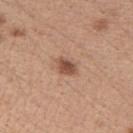The lesion was tiled from a total-body skin photograph and was not biopsied.
This is a white-light tile.
From the right upper arm.
A 15 mm close-up extracted from a 3D total-body photography capture.
The subject is a female about 45 years old.